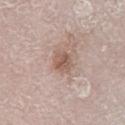workup: catalogued during a skin exam; not biopsied | location: the right lower leg | image: ~15 mm tile from a whole-body skin photo | patient: male, aged 78 to 82.